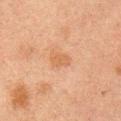No biopsy was performed on this lesion — it was imaged during a full skin examination and was not determined to be concerning. The patient is a male roughly 50 years of age. Located on the right upper arm. This is a cross-polarized tile. A 15 mm close-up extracted from a 3D total-body photography capture. The lesion-visualizer software estimated roughly 6 lightness units darker than nearby skin and a normalized border contrast of about 5.5. The software also gave a border-irregularity rating of about 3/10, a within-lesion color-variation index near 1.5/10, and peripheral color asymmetry of about 0.5. The analysis additionally found a lesion-detection confidence of about 100/100. The recorded lesion diameter is about 3 mm.